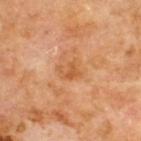| feature | finding |
|---|---|
| follow-up | total-body-photography surveillance lesion; no biopsy |